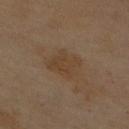Assessment:
Imaged during a routine full-body skin examination; the lesion was not biopsied and no histopathology is available.
Acquisition and patient details:
From the right upper arm. Automated image analysis of the tile measured a border-irregularity rating of about 3/10 and internal color variation of about 2 on a 0–10 scale. And it measured a nevus-likeness score of about 5/100. A female subject roughly 60 years of age. A close-up tile cropped from a whole-body skin photograph, about 15 mm across. This is a cross-polarized tile. Longest diameter approximately 3.5 mm.